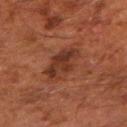Impression: Recorded during total-body skin imaging; not selected for excision or biopsy. Context: A region of skin cropped from a whole-body photographic capture, roughly 15 mm wide. About 5 mm across. An algorithmic analysis of the crop reported a lesion color around L≈29 a*≈21 b*≈25 in CIELAB, a lesion–skin lightness drop of about 7, and a lesion-to-skin contrast of about 7.5 (normalized; higher = more distinct). The analysis additionally found a border-irregularity index near 2.5/10, a within-lesion color-variation index near 4.5/10, and a peripheral color-asymmetry measure near 1.5. It also reported a classifier nevus-likeness of about 85/100 and a lesion-detection confidence of about 100/100. This is a cross-polarized tile. A male patient aged approximately 60. Located on the right forearm.